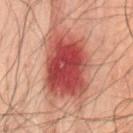The lesion was tiled from a total-body skin photograph and was not biopsied. A 15 mm crop from a total-body photograph taken for skin-cancer surveillance. On the lower back. This is a cross-polarized tile. A male patient, about 50 years old. Approximately 7.5 mm at its widest.